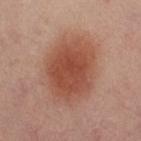<record>
<biopsy_status>not biopsied; imaged during a skin examination</biopsy_status>
<image>
  <source>total-body photography crop</source>
  <field_of_view_mm>15</field_of_view_mm>
</image>
<lesion_size>
  <long_diameter_mm_approx>7.5</long_diameter_mm_approx>
</lesion_size>
<patient>
  <sex>female</sex>
  <age_approx>40</age_approx>
</patient>
<lighting>cross-polarized</lighting>
<automated_metrics>
  <color_variation_0_10>4.0</color_variation_0_10>
  <peripheral_color_asymmetry>1.0</peripheral_color_asymmetry>
</automated_metrics>
<site>right thigh</site>
</record>